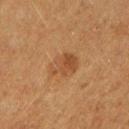<record>
  <biopsy_status>not biopsied; imaged during a skin examination</biopsy_status>
  <site>right forearm</site>
  <image>
    <source>total-body photography crop</source>
    <field_of_view_mm>15</field_of_view_mm>
  </image>
  <lighting>cross-polarized</lighting>
  <patient>
    <sex>female</sex>
    <age_approx>40</age_approx>
  </patient>
  <lesion_size>
    <long_diameter_mm_approx>3.5</long_diameter_mm_approx>
  </lesion_size>
</record>